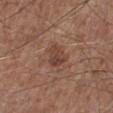Recorded during total-body skin imaging; not selected for excision or biopsy.
On the leg.
The lesion's longest dimension is about 3 mm.
A lesion tile, about 15 mm wide, cut from a 3D total-body photograph.
A male subject, in their 60s.
Automated image analysis of the tile measured a lesion area of about 5 mm² and a shape eccentricity near 0.7. The analysis additionally found an automated nevus-likeness rating near 15 out of 100 and a detector confidence of about 100 out of 100 that the crop contains a lesion.
This is a white-light tile.